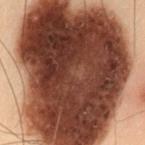{
  "lighting": "cross-polarized",
  "site": "left thigh",
  "image": {
    "source": "total-body photography crop",
    "field_of_view_mm": 15
  },
  "automated_metrics": {
    "eccentricity": 0.6,
    "shape_asymmetry": 0.25,
    "cielab_L": 31,
    "cielab_a": 20,
    "cielab_b": 23,
    "vs_skin_darker_L": 27.0,
    "vs_skin_contrast_norm": 20.5,
    "color_variation_0_10": 7.5,
    "peripheral_color_asymmetry": 3.0,
    "lesion_detection_confidence_0_100": 100
  },
  "lesion_size": {
    "long_diameter_mm_approx": 15.5
  },
  "patient": {
    "sex": "male",
    "age_approx": 55
  }
}